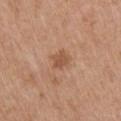site — the right upper arm; illumination — white-light; image source — ~15 mm crop, total-body skin-cancer survey; diameter — ~2.5 mm (longest diameter); patient — female, aged 38–42.